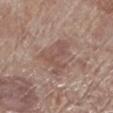Recorded during total-body skin imaging; not selected for excision or biopsy. Imaged with white-light lighting. Measured at roughly 5.5 mm in maximum diameter. From the left lower leg. A male patient about 70 years old. This image is a 15 mm lesion crop taken from a total-body photograph.